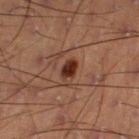Q: Was a biopsy performed?
A: total-body-photography surveillance lesion; no biopsy
Q: Lesion location?
A: the right thigh
Q: What kind of image is this?
A: ~15 mm tile from a whole-body skin photo
Q: Patient demographics?
A: male, roughly 55 years of age